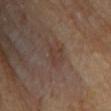Impression:
Recorded during total-body skin imaging; not selected for excision or biopsy.
Image and clinical context:
Captured under cross-polarized illumination. The subject is a male aged around 85 or older. The lesion is on the chest. A 15 mm close-up tile from a total-body photography series done for melanoma screening.